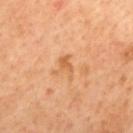| key | value |
|---|---|
| biopsy status | total-body-photography surveillance lesion; no biopsy |
| automated metrics | a mean CIELAB color near L≈60 a*≈24 b*≈41, a lesion–skin lightness drop of about 8, and a normalized border contrast of about 6 |
| subject | male, about 60 years old |
| lighting | cross-polarized illumination |
| anatomic site | the mid back |
| imaging modality | total-body-photography crop, ~15 mm field of view |